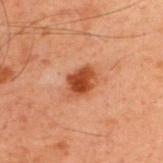No biopsy was performed on this lesion — it was imaged during a full skin examination and was not determined to be concerning. A 15 mm close-up extracted from a 3D total-body photography capture. The recorded lesion diameter is about 3.5 mm. A male subject aged 58–62. Automated tile analysis of the lesion measured a footprint of about 7 mm². And it measured an average lesion color of about L≈37 a*≈25 b*≈31 (CIELAB) and a lesion-to-skin contrast of about 10 (normalized; higher = more distinct). The analysis additionally found border irregularity of about 2 on a 0–10 scale and a within-lesion color-variation index near 4/10. On the upper back. Imaged with cross-polarized lighting.